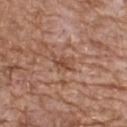workup: imaged on a skin check; not biopsied
anatomic site: the chest
subject: male, aged around 60
acquisition: ~15 mm crop, total-body skin-cancer survey
lighting: white-light